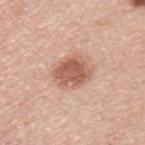{
  "patient": {
    "sex": "male",
    "age_approx": 45
  },
  "site": "upper back",
  "image": {
    "source": "total-body photography crop",
    "field_of_view_mm": 15
  }
}